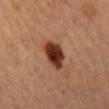The lesion was tiled from a total-body skin photograph and was not biopsied. The total-body-photography lesion software estimated a lesion area of about 9 mm² and a shape eccentricity near 0.85. The analysis additionally found radial color variation of about 1. The analysis additionally found a classifier nevus-likeness of about 100/100 and a lesion-detection confidence of about 100/100. This is a cross-polarized tile. Cropped from a total-body skin-imaging series; the visible field is about 15 mm. The patient is a female about 60 years old. From the mid back. The recorded lesion diameter is about 4.5 mm.